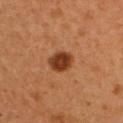On the upper back. A 15 mm close-up tile from a total-body photography series done for melanoma screening. The subject is a female in their 40s. Captured under cross-polarized illumination.